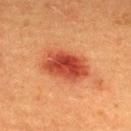Impression: The lesion was tiled from a total-body skin photograph and was not biopsied. Context: A lesion tile, about 15 mm wide, cut from a 3D total-body photograph. The lesion-visualizer software estimated an area of roughly 16 mm², a shape eccentricity near 0.8, and a shape-asymmetry score of about 0.15 (0 = symmetric). It also reported an automated nevus-likeness rating near 100 out of 100 and lesion-presence confidence of about 100/100. Imaged with cross-polarized lighting. A male patient aged around 40. Longest diameter approximately 6 mm. The lesion is located on the upper back.